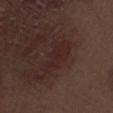notes — imaged on a skin check; not biopsied | location — the right thigh | acquisition — ~15 mm tile from a whole-body skin photo | patient — male, roughly 70 years of age | automated metrics — a lesion color around L≈24 a*≈18 b*≈18 in CIELAB and about 4 CIELAB-L* units darker than the surrounding skin.